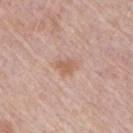Q: Is there a histopathology result?
A: catalogued during a skin exam; not biopsied
Q: Who is the patient?
A: male, approximately 75 years of age
Q: Automated lesion metrics?
A: a mean CIELAB color near L≈60 a*≈20 b*≈29 and a normalized lesion–skin contrast near 6.5; border irregularity of about 3 on a 0–10 scale, a color-variation rating of about 1/10, and a peripheral color-asymmetry measure near 0.5; an automated nevus-likeness rating near 10 out of 100 and lesion-presence confidence of about 100/100
Q: What is the anatomic site?
A: the arm
Q: What is the lesion's diameter?
A: ≈2.5 mm
Q: What lighting was used for the tile?
A: white-light illumination
Q: What kind of image is this?
A: ~15 mm crop, total-body skin-cancer survey